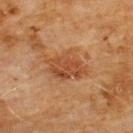The lesion's longest dimension is about 5 mm.
Imaged with cross-polarized lighting.
Automated image analysis of the tile measured about 9 CIELAB-L* units darker than the surrounding skin and a normalized border contrast of about 7. The software also gave an automated nevus-likeness rating near 25 out of 100 and lesion-presence confidence of about 100/100.
A close-up tile cropped from a whole-body skin photograph, about 15 mm across.
A male subject, aged around 60.
Located on the chest.